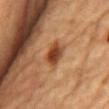notes: catalogued during a skin exam; not biopsied
subject: male, roughly 85 years of age or older
anatomic site: the front of the torso
lesion diameter: ≈3 mm
automated metrics: a lesion color around L≈37 a*≈22 b*≈32 in CIELAB and a lesion-to-skin contrast of about 10.5 (normalized; higher = more distinct)
tile lighting: cross-polarized illumination
image source: total-body-photography crop, ~15 mm field of view